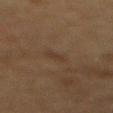Q: Was a biopsy performed?
A: imaged on a skin check; not biopsied
Q: What are the patient's age and sex?
A: female, aged 53–57
Q: What is the imaging modality?
A: ~15 mm crop, total-body skin-cancer survey
Q: What lighting was used for the tile?
A: cross-polarized illumination
Q: Where on the body is the lesion?
A: the mid back